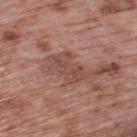This lesion was catalogued during total-body skin photography and was not selected for biopsy. Measured at roughly 4.5 mm in maximum diameter. This image is a 15 mm lesion crop taken from a total-body photograph. A male patient, roughly 70 years of age. Located on the upper back.